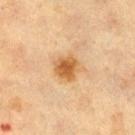This lesion was catalogued during total-body skin photography and was not selected for biopsy. The lesion is located on the right thigh. A female subject, roughly 40 years of age. This is a cross-polarized tile. Automated tile analysis of the lesion measured a lesion color around L≈53 a*≈19 b*≈38 in CIELAB, roughly 11 lightness units darker than nearby skin, and a normalized border contrast of about 8.5. It also reported a border-irregularity rating of about 2.5/10 and peripheral color asymmetry of about 1. The recorded lesion diameter is about 3.5 mm. A 15 mm crop from a total-body photograph taken for skin-cancer surveillance.